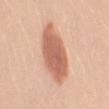The lesion was tiled from a total-body skin photograph and was not biopsied. A female patient about 25 years old. Measured at roughly 6.5 mm in maximum diameter. This is a white-light tile. A 15 mm close-up extracted from a 3D total-body photography capture. The lesion is on the left upper arm.